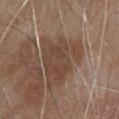{"biopsy_status": "not biopsied; imaged during a skin examination", "patient": {"sex": "male", "age_approx": 80}, "lesion_size": {"long_diameter_mm_approx": 7.0}, "site": "front of the torso", "image": {"source": "total-body photography crop", "field_of_view_mm": 15}}